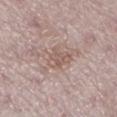Q: Was this lesion biopsied?
A: no biopsy performed (imaged during a skin exam)
Q: How was the tile lit?
A: white-light
Q: What kind of image is this?
A: 15 mm crop, total-body photography
Q: What is the lesion's diameter?
A: ≈3 mm
Q: Patient demographics?
A: female, aged 48 to 52
Q: Where on the body is the lesion?
A: the left lower leg